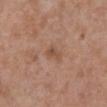{
  "biopsy_status": "not biopsied; imaged during a skin examination",
  "image": {
    "source": "total-body photography crop",
    "field_of_view_mm": 15
  },
  "lighting": "white-light",
  "site": "abdomen",
  "patient": {
    "sex": "male",
    "age_approx": 70
  }
}